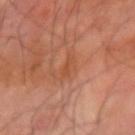The lesion was tiled from a total-body skin photograph and was not biopsied. An algorithmic analysis of the crop reported a border-irregularity index near 4/10, internal color variation of about 2 on a 0–10 scale, and a peripheral color-asymmetry measure near 0.5. And it measured a nevus-likeness score of about 0/100 and a lesion-detection confidence of about 100/100. This is a cross-polarized tile. The lesion is located on the right forearm. A roughly 15 mm field-of-view crop from a total-body skin photograph. The lesion's longest dimension is about 3 mm. A male subject, aged 68–72.